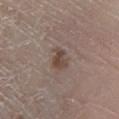Impression:
Captured during whole-body skin photography for melanoma surveillance; the lesion was not biopsied.
Background:
From the right lower leg. A male patient, about 65 years old. The total-body-photography lesion software estimated two-axis asymmetry of about 0.3. And it measured a lesion color around L≈44 a*≈15 b*≈23 in CIELAB, roughly 9 lightness units darker than nearby skin, and a normalized border contrast of about 7.5. The software also gave a color-variation rating of about 3.5/10 and radial color variation of about 1. A close-up tile cropped from a whole-body skin photograph, about 15 mm across. The tile uses white-light illumination.